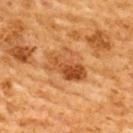<record>
  <biopsy_status>not biopsied; imaged during a skin examination</biopsy_status>
  <lesion_size>
    <long_diameter_mm_approx>5.0</long_diameter_mm_approx>
  </lesion_size>
  <automated_metrics>
    <border_irregularity_0_10>4.5</border_irregularity_0_10>
    <peripheral_color_asymmetry>2.5</peripheral_color_asymmetry>
    <nevus_likeness_0_100>5</nevus_likeness_0_100>
    <lesion_detection_confidence_0_100>100</lesion_detection_confidence_0_100>
  </automated_metrics>
  <image>
    <source>total-body photography crop</source>
    <field_of_view_mm>15</field_of_view_mm>
  </image>
  <patient>
    <sex>male</sex>
    <age_approx>60</age_approx>
  </patient>
  <lighting>cross-polarized</lighting>
  <site>back</site>
</record>